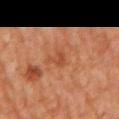Clinical impression: Captured during whole-body skin photography for melanoma surveillance; the lesion was not biopsied.